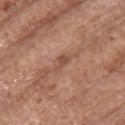Impression: This lesion was catalogued during total-body skin photography and was not selected for biopsy. Clinical summary: On the upper back. A female subject aged around 65. A 15 mm close-up tile from a total-body photography series done for melanoma screening.